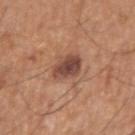follow-up: total-body-photography surveillance lesion; no biopsy | acquisition: ~15 mm tile from a whole-body skin photo | body site: the right upper arm | tile lighting: white-light illumination | lesion diameter: about 3.5 mm | subject: male, in their mid- to late 60s.